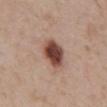Recorded during total-body skin imaging; not selected for excision or biopsy.